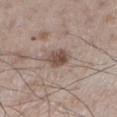Captured during whole-body skin photography for melanoma surveillance; the lesion was not biopsied. Imaged with white-light lighting. The total-body-photography lesion software estimated a lesion area of about 5 mm² and an eccentricity of roughly 0.55. The analysis additionally found a border-irregularity index near 1.5/10, internal color variation of about 3.5 on a 0–10 scale, and peripheral color asymmetry of about 1.5. From the left lower leg. A male subject aged approximately 60. A lesion tile, about 15 mm wide, cut from a 3D total-body photograph. The recorded lesion diameter is about 2.5 mm.